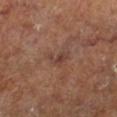Clinical impression: Captured during whole-body skin photography for melanoma surveillance; the lesion was not biopsied. Context: A 15 mm close-up tile from a total-body photography series done for melanoma screening. The tile uses cross-polarized illumination. The patient is a male aged approximately 60. From the leg. Approximately 2.5 mm at its widest. The total-body-photography lesion software estimated a mean CIELAB color near L≈38 a*≈19 b*≈24 and a lesion–skin lightness drop of about 7.